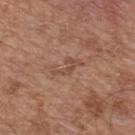Clinical impression: Imaged during a routine full-body skin examination; the lesion was not biopsied and no histopathology is available. Clinical summary: A male patient, aged 63–67. Cropped from a whole-body photographic skin survey; the tile spans about 15 mm. The recorded lesion diameter is about 3.5 mm. Captured under white-light illumination. An algorithmic analysis of the crop reported an area of roughly 3.5 mm² and an eccentricity of roughly 0.9. And it measured an average lesion color of about L≈48 a*≈21 b*≈28 (CIELAB), roughly 7 lightness units darker than nearby skin, and a lesion-to-skin contrast of about 5.5 (normalized; higher = more distinct). The software also gave a border-irregularity rating of about 8.5/10, a within-lesion color-variation index near 0/10, and radial color variation of about 0. Located on the upper back.